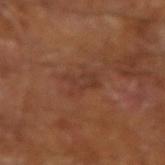The lesion was tiled from a total-body skin photograph and was not biopsied. The patient is a male in their mid-60s. Imaged with cross-polarized lighting. Approximately 3.5 mm at its widest. A close-up tile cropped from a whole-body skin photograph, about 15 mm across.